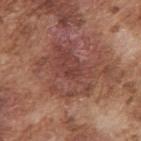| key | value |
|---|---|
| workup | total-body-photography surveillance lesion; no biopsy |
| tile lighting | white-light illumination |
| imaging modality | ~15 mm crop, total-body skin-cancer survey |
| subject | male, roughly 75 years of age |
| location | the right upper arm |
| diameter | ≈7.5 mm |